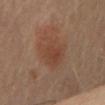No biopsy was performed on this lesion — it was imaged during a full skin examination and was not determined to be concerning. A male patient, about 85 years old or older. The tile uses cross-polarized illumination. Automated tile analysis of the lesion measured lesion-presence confidence of about 100/100. This image is a 15 mm lesion crop taken from a total-body photograph. Measured at roughly 5 mm in maximum diameter. Located on the chest.